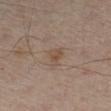Part of a total-body skin-imaging series; this lesion was reviewed on a skin check and was not flagged for biopsy.
Measured at roughly 2.5 mm in maximum diameter.
The total-body-photography lesion software estimated a lesion area of about 3.5 mm², an outline eccentricity of about 0.7 (0 = round, 1 = elongated), and two-axis asymmetry of about 0.3. The analysis additionally found border irregularity of about 2.5 on a 0–10 scale, internal color variation of about 3 on a 0–10 scale, and a peripheral color-asymmetry measure near 1. And it measured a lesion-detection confidence of about 100/100.
A male patient, about 50 years old.
From the left leg.
Cropped from a whole-body photographic skin survey; the tile spans about 15 mm.
The tile uses cross-polarized illumination.